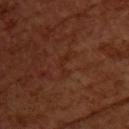The lesion was photographed on a routine skin check and not biopsied; there is no pathology result.